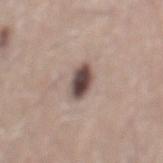Q: Was a biopsy performed?
A: total-body-photography surveillance lesion; no biopsy
Q: What is the imaging modality?
A: ~15 mm crop, total-body skin-cancer survey
Q: Where on the body is the lesion?
A: the mid back
Q: How was the tile lit?
A: white-light illumination
Q: Patient demographics?
A: male, roughly 75 years of age